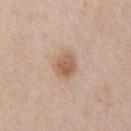Clinical impression:
Part of a total-body skin-imaging series; this lesion was reviewed on a skin check and was not flagged for biopsy.
Background:
A male subject aged 58–62. Cropped from a whole-body photographic skin survey; the tile spans about 15 mm. The lesion-visualizer software estimated a footprint of about 6 mm², an outline eccentricity of about 0.65 (0 = round, 1 = elongated), and a symmetry-axis asymmetry near 0.2. The software also gave a within-lesion color-variation index near 3/10 and peripheral color asymmetry of about 1. Captured under white-light illumination. Longest diameter approximately 3 mm. From the chest.